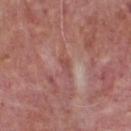workup = catalogued during a skin exam; not biopsied | patient = male, in their mid-70s | image source = 15 mm crop, total-body photography | body site = the front of the torso.